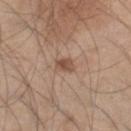The lesion was photographed on a routine skin check and not biopsied; there is no pathology result.